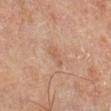Impression:
Captured during whole-body skin photography for melanoma surveillance; the lesion was not biopsied.
Acquisition and patient details:
Located on the right lower leg. A male patient, in their mid- to late 60s. A region of skin cropped from a whole-body photographic capture, roughly 15 mm wide.